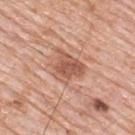<record>
<automated_metrics>
  <area_mm2_approx>8.5</area_mm2_approx>
  <eccentricity>0.45</eccentricity>
  <shape_asymmetry>0.4</shape_asymmetry>
  <cielab_L>56</cielab_L>
  <cielab_a>24</cielab_a>
  <cielab_b>30</cielab_b>
  <vs_skin_contrast_norm>7.5</vs_skin_contrast_norm>
</automated_metrics>
<patient>
  <sex>male</sex>
  <age_approx>80</age_approx>
</patient>
<site>back</site>
<image>
  <source>total-body photography crop</source>
  <field_of_view_mm>15</field_of_view_mm>
</image>
<lesion_size>
  <long_diameter_mm_approx>4.0</long_diameter_mm_approx>
</lesion_size>
</record>